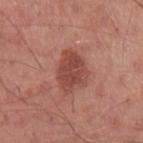notes: imaged on a skin check; not biopsied | patient: male, aged 28–32 | image: ~15 mm tile from a whole-body skin photo | lighting: white-light | site: the arm.